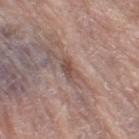Clinical impression: This lesion was catalogued during total-body skin photography and was not selected for biopsy. Background: The patient is a female roughly 65 years of age. Imaged with white-light lighting. The lesion is located on the right thigh. The lesion-visualizer software estimated a lesion area of about 3.5 mm² and a shape eccentricity near 0.85. The analysis additionally found a lesion–skin lightness drop of about 10 and a normalized lesion–skin contrast near 7.5. And it measured border irregularity of about 3 on a 0–10 scale and a peripheral color-asymmetry measure near 0.5. A region of skin cropped from a whole-body photographic capture, roughly 15 mm wide.